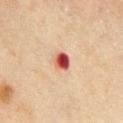Q: Was a biopsy performed?
A: no biopsy performed (imaged during a skin exam)
Q: Patient demographics?
A: male, aged approximately 70
Q: What kind of image is this?
A: ~15 mm tile from a whole-body skin photo
Q: Lesion location?
A: the chest